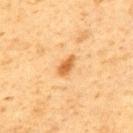Assessment: The lesion was tiled from a total-body skin photograph and was not biopsied. Context: The lesion is on the upper back. The recorded lesion diameter is about 2.5 mm. Cropped from a whole-body photographic skin survey; the tile spans about 15 mm. This is a cross-polarized tile. A male patient, aged approximately 60.